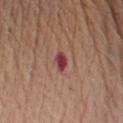- workup — catalogued during a skin exam; not biopsied
- subject — male, approximately 70 years of age
- acquisition — 15 mm crop, total-body photography
- location — the left upper arm
- size — ~2.5 mm (longest diameter)
- lighting — white-light illumination